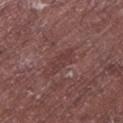{
  "biopsy_status": "not biopsied; imaged during a skin examination",
  "lighting": "white-light",
  "patient": {
    "sex": "male",
    "age_approx": 65
  },
  "image": {
    "source": "total-body photography crop",
    "field_of_view_mm": 15
  },
  "lesion_size": {
    "long_diameter_mm_approx": 4.0
  },
  "site": "left lower leg"
}